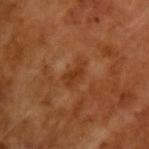| key | value |
|---|---|
| biopsy status | imaged on a skin check; not biopsied |
| patient | male, aged 63–67 |
| lesion diameter | ≈3.5 mm |
| image-analysis metrics | an average lesion color of about L≈36 a*≈25 b*≈35 (CIELAB), about 6 CIELAB-L* units darker than the surrounding skin, and a normalized border contrast of about 6; a border-irregularity index near 3.5/10, a color-variation rating of about 1.5/10, and a peripheral color-asymmetry measure near 0.5 |
| illumination | cross-polarized illumination |
| image source | 15 mm crop, total-body photography |